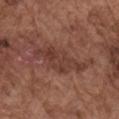Assessment: Imaged during a routine full-body skin examination; the lesion was not biopsied and no histopathology is available. Background: A male patient, about 75 years old. A region of skin cropped from a whole-body photographic capture, roughly 15 mm wide. On the right upper arm.